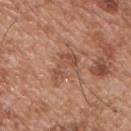follow-up=catalogued during a skin exam; not biopsied | subject=male, approximately 55 years of age | illumination=white-light | anatomic site=the front of the torso | acquisition=~15 mm tile from a whole-body skin photo.